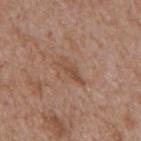– follow-up · imaged on a skin check; not biopsied
– lesion diameter · about 4 mm
– image · ~15 mm crop, total-body skin-cancer survey
– illumination · white-light
– TBP lesion metrics · two-axis asymmetry of about 0.35; an average lesion color of about L≈49 a*≈20 b*≈29 (CIELAB), a lesion–skin lightness drop of about 7, and a normalized lesion–skin contrast near 6; a color-variation rating of about 2.5/10 and a peripheral color-asymmetry measure near 0.5; a nevus-likeness score of about 0/100 and a lesion-detection confidence of about 95/100
– subject · male, in their mid- to late 60s
– site · the mid back